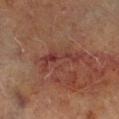{"biopsy_status": "not biopsied; imaged during a skin examination", "patient": {"sex": "male", "age_approx": 70}, "site": "right lower leg", "lighting": "cross-polarized", "image": {"source": "total-body photography crop", "field_of_view_mm": 15}, "lesion_size": {"long_diameter_mm_approx": 6.0}}